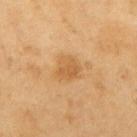- follow-up — catalogued during a skin exam; not biopsied
- acquisition — ~15 mm crop, total-body skin-cancer survey
- site — the right upper arm
- lesion size — ~3 mm (longest diameter)
- automated metrics — a mean CIELAB color near L≈59 a*≈20 b*≈42, about 8 CIELAB-L* units darker than the surrounding skin, and a normalized lesion–skin contrast near 6; an automated nevus-likeness rating near 40 out of 100 and a detector confidence of about 100 out of 100 that the crop contains a lesion
- subject — male, approximately 60 years of age
- tile lighting — cross-polarized